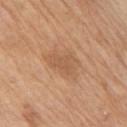This lesion was catalogued during total-body skin photography and was not selected for biopsy. The lesion-visualizer software estimated a lesion color around L≈56 a*≈20 b*≈34 in CIELAB. It also reported a border-irregularity rating of about 4/10, internal color variation of about 2 on a 0–10 scale, and a peripheral color-asymmetry measure near 0.5. The subject is a male aged 68–72. The tile uses white-light illumination. Approximately 3.5 mm at its widest. From the right upper arm. Cropped from a whole-body photographic skin survey; the tile spans about 15 mm.